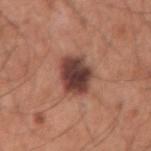| feature | finding |
|---|---|
| biopsy status | catalogued during a skin exam; not biopsied |
| acquisition | total-body-photography crop, ~15 mm field of view |
| patient | male, aged around 60 |
| size | ≈4 mm |
| anatomic site | the right upper arm |